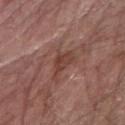The lesion was tiled from a total-body skin photograph and was not biopsied. A lesion tile, about 15 mm wide, cut from a 3D total-body photograph. A male patient roughly 65 years of age. This is a white-light tile. Longest diameter approximately 3.5 mm. From the right forearm. An algorithmic analysis of the crop reported an average lesion color of about L≈41 a*≈22 b*≈24 (CIELAB), roughly 8 lightness units darker than nearby skin, and a normalized lesion–skin contrast near 6.5. The software also gave lesion-presence confidence of about 100/100.